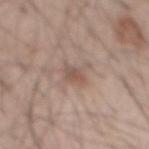notes: imaged on a skin check; not biopsied | patient: male, about 50 years old | image: 15 mm crop, total-body photography | body site: the back | lesion size: about 3 mm | lighting: white-light.